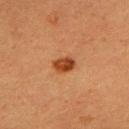<lesion>
<biopsy_status>not biopsied; imaged during a skin examination</biopsy_status>
<automated_metrics>
  <eccentricity>0.7</eccentricity>
  <shape_asymmetry>0.25</shape_asymmetry>
  <vs_skin_darker_L>12.0</vs_skin_darker_L>
  <vs_skin_contrast_norm>10.0</vs_skin_contrast_norm>
</automated_metrics>
<image>
  <source>total-body photography crop</source>
  <field_of_view_mm>15</field_of_view_mm>
</image>
<site>upper back</site>
<lesion_size>
  <long_diameter_mm_approx>2.5</long_diameter_mm_approx>
</lesion_size>
<lighting>cross-polarized</lighting>
<patient>
  <sex>male</sex>
  <age_approx>40</age_approx>
</patient>
</lesion>